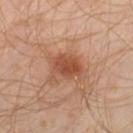notes: catalogued during a skin exam; not biopsied.